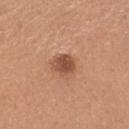Q: Is there a histopathology result?
A: imaged on a skin check; not biopsied
Q: Illumination type?
A: white-light
Q: Who is the patient?
A: male, in their mid- to late 30s
Q: Where on the body is the lesion?
A: the head or neck
Q: Lesion size?
A: ≈3 mm
Q: How was this image acquired?
A: 15 mm crop, total-body photography